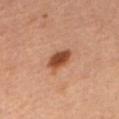follow-up: catalogued during a skin exam; not biopsied
anatomic site: the front of the torso
size: about 3.5 mm
acquisition: 15 mm crop, total-body photography
patient: female
illumination: cross-polarized